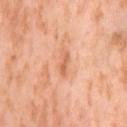Part of a total-body skin-imaging series; this lesion was reviewed on a skin check and was not flagged for biopsy. Imaged with cross-polarized lighting. Cropped from a whole-body photographic skin survey; the tile spans about 15 mm. Located on the right thigh. Longest diameter approximately 3.5 mm. The patient is a female aged approximately 55.